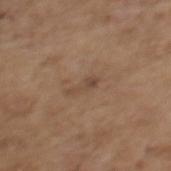{"biopsy_status": "not biopsied; imaged during a skin examination", "patient": {"sex": "female", "age_approx": 65}, "automated_metrics": {"vs_skin_contrast_norm": 6.0}, "lighting": "white-light", "site": "mid back", "image": {"source": "total-body photography crop", "field_of_view_mm": 15}}